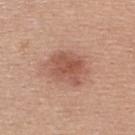Case summary:
- notes — total-body-photography surveillance lesion; no biopsy
- patient — female, approximately 30 years of age
- imaging modality — ~15 mm tile from a whole-body skin photo
- diameter — ~4.5 mm (longest diameter)
- automated lesion analysis — an area of roughly 13 mm², an eccentricity of roughly 0.55, and two-axis asymmetry of about 0.2; a mean CIELAB color near L≈54 a*≈24 b*≈29, about 10 CIELAB-L* units darker than the surrounding skin, and a lesion-to-skin contrast of about 7 (normalized; higher = more distinct); peripheral color asymmetry of about 1
- tile lighting — white-light
- location — the upper back